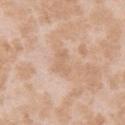Captured during whole-body skin photography for melanoma surveillance; the lesion was not biopsied. A female patient in their mid- to late 20s. A 15 mm crop from a total-body photograph taken for skin-cancer surveillance. About 3.5 mm across. On the right upper arm.